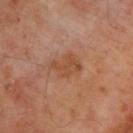biopsy status: total-body-photography surveillance lesion; no biopsy | imaging modality: 15 mm crop, total-body photography | site: the upper back | subject: male, approximately 65 years of age.